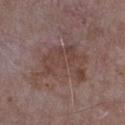The lesion was tiled from a total-body skin photograph and was not biopsied.
Imaged with white-light lighting.
The subject is a male aged around 65.
A 15 mm crop from a total-body photograph taken for skin-cancer surveillance.
From the left upper arm.
The recorded lesion diameter is about 8 mm.
Automated image analysis of the tile measured a border-irregularity index near 7.5/10, a within-lesion color-variation index near 3.5/10, and a peripheral color-asymmetry measure near 1.